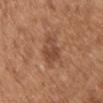Imaged with white-light lighting. Longest diameter approximately 4 mm. On the chest. A male subject, aged 63 to 67. A close-up tile cropped from a whole-body skin photograph, about 15 mm across.